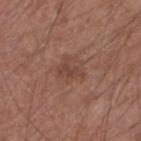Notes:
- patient · male, approximately 55 years of age
- illumination · white-light
- imaging modality · ~15 mm crop, total-body skin-cancer survey
- body site · the right forearm